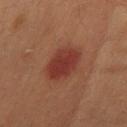– workup: imaged on a skin check; not biopsied
– location: the abdomen
– diameter: ≈4 mm
– image source: total-body-photography crop, ~15 mm field of view
– patient: male, aged approximately 50